A 15 mm close-up extracted from a 3D total-body photography capture · a female subject, aged around 60 · the tile uses white-light illumination · located on the head or neck.
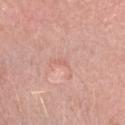On excision, pathology confirmed an intradermal melanocytic nevus.